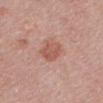Captured during whole-body skin photography for melanoma surveillance; the lesion was not biopsied. Located on the mid back. A region of skin cropped from a whole-body photographic capture, roughly 15 mm wide. The recorded lesion diameter is about 4 mm. A male patient approximately 50 years of age. The tile uses white-light illumination.